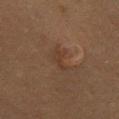Q: Is there a histopathology result?
A: imaged on a skin check; not biopsied
Q: What kind of image is this?
A: ~15 mm tile from a whole-body skin photo
Q: Lesion location?
A: the chest
Q: What are the patient's age and sex?
A: male, about 50 years old
Q: What lighting was used for the tile?
A: cross-polarized illumination
Q: What did automated image analysis measure?
A: a mean CIELAB color near L≈28 a*≈14 b*≈23, a lesion–skin lightness drop of about 5, and a normalized lesion–skin contrast near 5.5; border irregularity of about 6.5 on a 0–10 scale, internal color variation of about 0 on a 0–10 scale, and a peripheral color-asymmetry measure near 0